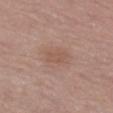biopsy_status: not biopsied; imaged during a skin examination
lighting: white-light
patient:
  sex: female
  age_approx: 55
site: left thigh
image:
  source: total-body photography crop
  field_of_view_mm: 15
lesion_size:
  long_diameter_mm_approx: 3.0
automated_metrics:
  area_mm2_approx: 5.5
  eccentricity: 0.8
  shape_asymmetry: 0.15